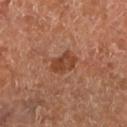The lesion was tiled from a total-body skin photograph and was not biopsied. From the leg. The patient is aged around 65. A lesion tile, about 15 mm wide, cut from a 3D total-body photograph. Captured under cross-polarized illumination. Automated tile analysis of the lesion measured a lesion color around L≈42 a*≈24 b*≈31 in CIELAB, roughly 9 lightness units darker than nearby skin, and a normalized border contrast of about 7.5. And it measured a border-irregularity rating of about 2/10, a within-lesion color-variation index near 3.5/10, and peripheral color asymmetry of about 1. The software also gave a nevus-likeness score of about 55/100 and a detector confidence of about 100 out of 100 that the crop contains a lesion. Measured at roughly 3.5 mm in maximum diameter.